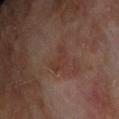Q: Is there a histopathology result?
A: total-body-photography surveillance lesion; no biopsy
Q: What is the imaging modality?
A: ~15 mm crop, total-body skin-cancer survey
Q: Where on the body is the lesion?
A: the upper back
Q: Illumination type?
A: cross-polarized
Q: What did automated image analysis measure?
A: border irregularity of about 5 on a 0–10 scale, a within-lesion color-variation index near 1/10, and radial color variation of about 0.5; a detector confidence of about 100 out of 100 that the crop contains a lesion
Q: What are the patient's age and sex?
A: male, roughly 70 years of age